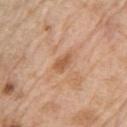Recorded during total-body skin imaging; not selected for excision or biopsy. This image is a 15 mm lesion crop taken from a total-body photograph. On the left upper arm. The lesion-visualizer software estimated a footprint of about 3 mm², an eccentricity of roughly 0.9, and two-axis asymmetry of about 0.35. The software also gave a mean CIELAB color near L≈57 a*≈22 b*≈36 and a lesion–skin lightness drop of about 10. The patient is a male aged approximately 70.